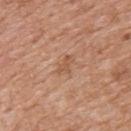Imaged during a routine full-body skin examination; the lesion was not biopsied and no histopathology is available. A 15 mm crop from a total-body photograph taken for skin-cancer surveillance. The lesion is on the upper back. The tile uses white-light illumination. The patient is a male aged 58–62. Automated tile analysis of the lesion measured a border-irregularity index near 5/10, a color-variation rating of about 0/10, and radial color variation of about 0. And it measured a lesion-detection confidence of about 100/100. Measured at roughly 2.5 mm in maximum diameter.